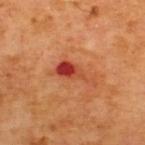workup: imaged on a skin check; not biopsied | acquisition: total-body-photography crop, ~15 mm field of view | patient: male, in their mid- to late 60s | body site: the upper back | automated lesion analysis: a lesion area of about 6.5 mm² and an outline eccentricity of about 0.9 (0 = round, 1 = elongated); roughly 12 lightness units darker than nearby skin and a normalized border contrast of about 9; a border-irregularity index near 6.5/10 and a within-lesion color-variation index near 9/10; a nevus-likeness score of about 0/100 and a detector confidence of about 100 out of 100 that the crop contains a lesion | lighting: cross-polarized illumination.